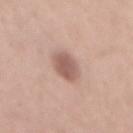Clinical summary:
About 4 mm across. A region of skin cropped from a whole-body photographic capture, roughly 15 mm wide. On the mid back. This is a white-light tile. Automated tile analysis of the lesion measured an area of roughly 7.5 mm² and an eccentricity of roughly 0.8. The analysis additionally found a nevus-likeness score of about 70/100. A male patient, roughly 30 years of age.